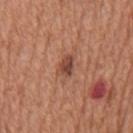This lesion was catalogued during total-body skin photography and was not selected for biopsy. On the mid back. About 3 mm across. The tile uses white-light illumination. A male subject, in their mid-60s. A lesion tile, about 15 mm wide, cut from a 3D total-body photograph.